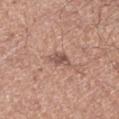Captured during whole-body skin photography for melanoma surveillance; the lesion was not biopsied.
A 15 mm close-up tile from a total-body photography series done for melanoma screening.
Measured at roughly 3 mm in maximum diameter.
The lesion is located on the right lower leg.
A male subject, aged around 50.
This is a white-light tile.